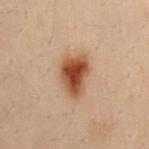<tbp_lesion>
  <biopsy_status>not biopsied; imaged during a skin examination</biopsy_status>
  <image>
    <source>total-body photography crop</source>
    <field_of_view_mm>15</field_of_view_mm>
  </image>
  <patient>
    <sex>male</sex>
    <age_approx>30</age_approx>
  </patient>
  <lesion_size>
    <long_diameter_mm_approx>4.5</long_diameter_mm_approx>
  </lesion_size>
  <site>mid back</site>
</tbp_lesion>